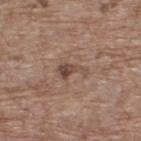Notes:
• biopsy status · imaged on a skin check; not biopsied
• acquisition · total-body-photography crop, ~15 mm field of view
• anatomic site · the back
• subject · male, aged approximately 70